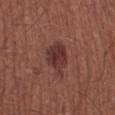* biopsy status — imaged on a skin check; not biopsied
* image-analysis metrics — border irregularity of about 3 on a 0–10 scale and internal color variation of about 4 on a 0–10 scale; a classifier nevus-likeness of about 45/100 and lesion-presence confidence of about 100/100
* size — ≈4 mm
* lighting — white-light
* subject — male, aged 63–67
* anatomic site — the right lower leg
* imaging modality — ~15 mm crop, total-body skin-cancer survey